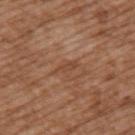workup: no biopsy performed (imaged during a skin exam)
lesion diameter: about 2.5 mm
subject: male, about 75 years old
anatomic site: the upper back
tile lighting: white-light illumination
image: ~15 mm crop, total-body skin-cancer survey